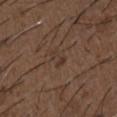Recorded during total-body skin imaging; not selected for excision or biopsy.
From the chest.
A roughly 15 mm field-of-view crop from a total-body skin photograph.
Approximately 2.5 mm at its widest.
A male subject, aged approximately 50.